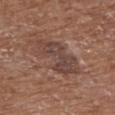biopsy status=no biopsy performed (imaged during a skin exam)
body site=the front of the torso
subject=female, in their mid- to late 70s
TBP lesion metrics=an area of roughly 16 mm², a shape eccentricity near 0.9, and two-axis asymmetry of about 0.3; an average lesion color of about L≈43 a*≈18 b*≈23 (CIELAB), roughly 8 lightness units darker than nearby skin, and a normalized border contrast of about 7; a border-irregularity rating of about 4.5/10
image=total-body-photography crop, ~15 mm field of view
lesion diameter=about 7 mm
lighting=white-light